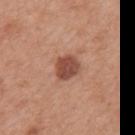<tbp_lesion>
<biopsy_status>not biopsied; imaged during a skin examination</biopsy_status>
<image>
  <source>total-body photography crop</source>
  <field_of_view_mm>15</field_of_view_mm>
</image>
<site>mid back</site>
<patient>
  <sex>male</sex>
  <age_approx>70</age_approx>
</patient>
</tbp_lesion>